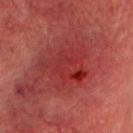Clinical impression:
Part of a total-body skin-imaging series; this lesion was reviewed on a skin check and was not flagged for biopsy.
Acquisition and patient details:
Cropped from a whole-body photographic skin survey; the tile spans about 15 mm. The tile uses cross-polarized illumination. The patient is a male aged around 70. About 5 mm across. From the head or neck.